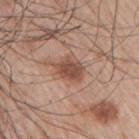biopsy status = imaged on a skin check; not biopsied | lesion diameter = ≈4 mm | anatomic site = the right upper arm | patient = male, approximately 65 years of age | lighting = white-light illumination | acquisition = total-body-photography crop, ~15 mm field of view | automated metrics = a classifier nevus-likeness of about 60/100 and a lesion-detection confidence of about 100/100.